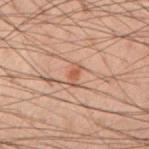biopsy status: imaged on a skin check; not biopsied
diameter: ~2.5 mm (longest diameter)
image source: ~15 mm tile from a whole-body skin photo
patient: male, aged 38–42
anatomic site: the right forearm